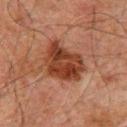Clinical impression:
Captured during whole-body skin photography for melanoma surveillance; the lesion was not biopsied.
Context:
The lesion is located on the chest. A male patient, aged 58 to 62. A lesion tile, about 15 mm wide, cut from a 3D total-body photograph. Automated image analysis of the tile measured about 11 CIELAB-L* units darker than the surrounding skin. Imaged with cross-polarized lighting.